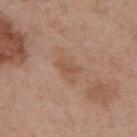The tile uses white-light illumination. The lesion is located on the back. About 3 mm across. A male subject about 55 years old. Cropped from a whole-body photographic skin survey; the tile spans about 15 mm. The total-body-photography lesion software estimated a border-irregularity rating of about 5.5/10 and radial color variation of about 0.